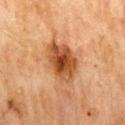Recorded during total-body skin imaging; not selected for excision or biopsy.
The tile uses cross-polarized illumination.
About 5.5 mm across.
A close-up tile cropped from a whole-body skin photograph, about 15 mm across.
The lesion is located on the mid back.
A male subject, in their 70s.
An algorithmic analysis of the crop reported an area of roughly 15 mm², a shape eccentricity near 0.75, and a shape-asymmetry score of about 0.2 (0 = symmetric). The software also gave a lesion color around L≈41 a*≈22 b*≈34 in CIELAB, roughly 13 lightness units darker than nearby skin, and a lesion-to-skin contrast of about 10.5 (normalized; higher = more distinct). It also reported a border-irregularity rating of about 2/10 and radial color variation of about 1.5. It also reported an automated nevus-likeness rating near 90 out of 100 and a detector confidence of about 100 out of 100 that the crop contains a lesion.